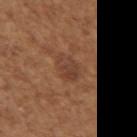Clinical impression:
Recorded during total-body skin imaging; not selected for excision or biopsy.
Clinical summary:
About 3 mm across. Cropped from a total-body skin-imaging series; the visible field is about 15 mm. The subject is a female in their mid-70s. Imaged with white-light lighting. On the left upper arm.